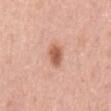follow-up — no biopsy performed (imaged during a skin exam); body site — the mid back; lighting — white-light illumination; imaging modality — 15 mm crop, total-body photography; automated lesion analysis — roughly 13 lightness units darker than nearby skin and a normalized lesion–skin contrast near 8.5; diameter — ~3 mm (longest diameter); subject — male, approximately 65 years of age.